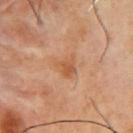Captured during whole-body skin photography for melanoma surveillance; the lesion was not biopsied. The lesion is on the chest. A male patient in their mid-50s. This image is a 15 mm lesion crop taken from a total-body photograph.